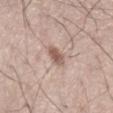This lesion was catalogued during total-body skin photography and was not selected for biopsy. Approximately 2.5 mm at its widest. This is a white-light tile. A male patient, aged 58 to 62. Cropped from a total-body skin-imaging series; the visible field is about 15 mm. Located on the abdomen.